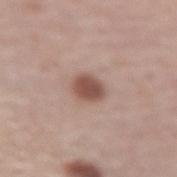A female patient, aged 58–62. Imaged with white-light lighting. On the left forearm. The total-body-photography lesion software estimated an average lesion color of about L≈51 a*≈20 b*≈24 (CIELAB), about 13 CIELAB-L* units darker than the surrounding skin, and a lesion-to-skin contrast of about 9 (normalized; higher = more distinct). It also reported an automated nevus-likeness rating near 95 out of 100 and a lesion-detection confidence of about 100/100. A region of skin cropped from a whole-body photographic capture, roughly 15 mm wide. About 3 mm across.